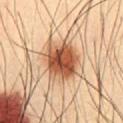- notes — imaged on a skin check; not biopsied
- location — the chest
- patient — male, aged 33 to 37
- lesion diameter — about 4.5 mm
- lighting — cross-polarized
- TBP lesion metrics — an area of roughly 15 mm², an eccentricity of roughly 0.4, and a shape-asymmetry score of about 0.15 (0 = symmetric); a border-irregularity rating of about 1.5/10 and a peripheral color-asymmetry measure near 2.5; a detector confidence of about 100 out of 100 that the crop contains a lesion
- acquisition — total-body-photography crop, ~15 mm field of view